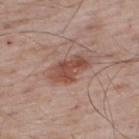follow-up — imaged on a skin check; not biopsied
image source — total-body-photography crop, ~15 mm field of view
location — the upper back
illumination — white-light illumination
patient — male, in their mid- to late 60s
TBP lesion metrics — an eccentricity of roughly 0.85 and a shape-asymmetry score of about 0.25 (0 = symmetric); border irregularity of about 3 on a 0–10 scale, internal color variation of about 3.5 on a 0–10 scale, and peripheral color asymmetry of about 1.5; an automated nevus-likeness rating near 75 out of 100
size — ≈4.5 mm